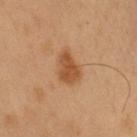Assessment: Part of a total-body skin-imaging series; this lesion was reviewed on a skin check and was not flagged for biopsy. Background: From the arm. An algorithmic analysis of the crop reported a footprint of about 7.5 mm² and a shape-asymmetry score of about 0.3 (0 = symmetric). The analysis additionally found lesion-presence confidence of about 100/100. The tile uses cross-polarized illumination. About 4 mm across. A male subject, about 60 years old. Cropped from a total-body skin-imaging series; the visible field is about 15 mm.